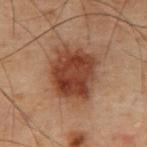No biopsy was performed on this lesion — it was imaged during a full skin examination and was not determined to be concerning.
Cropped from a total-body skin-imaging series; the visible field is about 15 mm.
A male subject roughly 70 years of age.
The lesion is on the chest.
This is a cross-polarized tile.